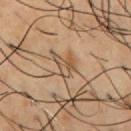– biopsy status · no biopsy performed (imaged during a skin exam)
– size · ≈3 mm
– location · the chest
– patient · male, aged around 55
– lighting · cross-polarized illumination
– image · ~15 mm tile from a whole-body skin photo